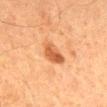{"biopsy_status": "not biopsied; imaged during a skin examination", "patient": {"sex": "male", "age_approx": 65}, "lighting": "cross-polarized", "site": "mid back", "lesion_size": {"long_diameter_mm_approx": 3.5}, "automated_metrics": {"cielab_L": 49, "cielab_a": 24, "cielab_b": 37, "vs_skin_contrast_norm": 8.0, "border_irregularity_0_10": 2.5, "peripheral_color_asymmetry": 1.5, "nevus_likeness_0_100": 90, "lesion_detection_confidence_0_100": 100}, "image": {"source": "total-body photography crop", "field_of_view_mm": 15}}